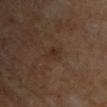biopsy status — imaged on a skin check; not biopsied
body site — the chest
automated lesion analysis — an average lesion color of about L≈28 a*≈15 b*≈24 (CIELAB) and a lesion–skin lightness drop of about 5
subject — male, aged approximately 70
image source — ~15 mm crop, total-body skin-cancer survey